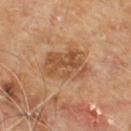The lesion was photographed on a routine skin check and not biopsied; there is no pathology result.
The tile uses cross-polarized illumination.
A male patient, aged 58 to 62.
An algorithmic analysis of the crop reported an average lesion color of about L≈49 a*≈20 b*≈34 (CIELAB) and a lesion-to-skin contrast of about 7 (normalized; higher = more distinct). The analysis additionally found border irregularity of about 3 on a 0–10 scale, internal color variation of about 5.5 on a 0–10 scale, and a peripheral color-asymmetry measure near 2.
The lesion's longest dimension is about 5.5 mm.
On the upper back.
A lesion tile, about 15 mm wide, cut from a 3D total-body photograph.